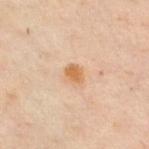{
  "biopsy_status": "not biopsied; imaged during a skin examination",
  "automated_metrics": {
    "cielab_L": 52,
    "cielab_a": 18,
    "cielab_b": 33,
    "vs_skin_contrast_norm": 8.5,
    "nevus_likeness_0_100": 80,
    "lesion_detection_confidence_0_100": 100
  },
  "lesion_size": {
    "long_diameter_mm_approx": 2.5
  },
  "image": {
    "source": "total-body photography crop",
    "field_of_view_mm": 15
  },
  "patient": {
    "sex": "male",
    "age_approx": 50
  },
  "site": "chest",
  "lighting": "cross-polarized"
}